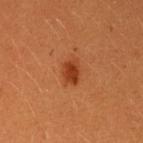follow-up: imaged on a skin check; not biopsied | lesion size: ≈3 mm | patient: female, about 30 years old | anatomic site: the left upper arm | acquisition: total-body-photography crop, ~15 mm field of view | lighting: cross-polarized illumination.